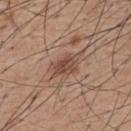workup: imaged on a skin check; not biopsied
size: ≈4.5 mm
illumination: white-light illumination
site: the upper back
TBP lesion metrics: an area of roughly 9 mm², an eccentricity of roughly 0.8, and a shape-asymmetry score of about 0.3 (0 = symmetric); a detector confidence of about 100 out of 100 that the crop contains a lesion
image: 15 mm crop, total-body photography
patient: male, roughly 55 years of age